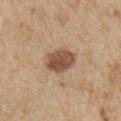workup: no biopsy performed (imaged during a skin exam)
image: ~15 mm tile from a whole-body skin photo
subject: male, aged approximately 70
location: the left upper arm
lighting: white-light